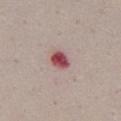The lesion was tiled from a total-body skin photograph and was not biopsied.
A roughly 15 mm field-of-view crop from a total-body skin photograph.
From the front of the torso.
The patient is a female aged around 50.